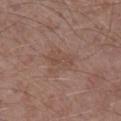Findings:
- workup — total-body-photography surveillance lesion; no biopsy
- anatomic site — the left lower leg
- patient — male, in their mid- to late 40s
- image — 15 mm crop, total-body photography